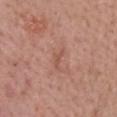Findings:
– notes · catalogued during a skin exam; not biopsied
– body site · the head or neck
– image-analysis metrics · a lesion color around L≈53 a*≈23 b*≈28 in CIELAB, a lesion–skin lightness drop of about 7, and a normalized lesion–skin contrast near 5; internal color variation of about 0 on a 0–10 scale and a peripheral color-asymmetry measure near 0; an automated nevus-likeness rating near 0 out of 100 and a detector confidence of about 100 out of 100 that the crop contains a lesion
– subject · male, aged 38–42
– illumination · white-light
– image · total-body-photography crop, ~15 mm field of view